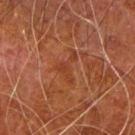Impression:
Recorded during total-body skin imaging; not selected for excision or biopsy.
Context:
A male patient, in their 80s. On the arm. A lesion tile, about 15 mm wide, cut from a 3D total-body photograph. Approximately 4 mm at its widest.